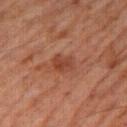No biopsy was performed on this lesion — it was imaged during a full skin examination and was not determined to be concerning. The tile uses cross-polarized illumination. This image is a 15 mm lesion crop taken from a total-body photograph. About 2.5 mm across. From the leg. The subject is a male aged around 60.